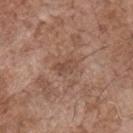Notes:
• biopsy status · no biopsy performed (imaged during a skin exam)
• location · the chest
• acquisition · ~15 mm crop, total-body skin-cancer survey
• subject · male, aged around 70